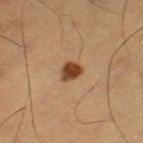follow-up = imaged on a skin check; not biopsied
location = the left thigh
image = 15 mm crop, total-body photography
illumination = cross-polarized illumination
patient = male, aged 58 to 62
automated metrics = a shape eccentricity near 0.6 and two-axis asymmetry of about 0.3; lesion-presence confidence of about 100/100
lesion diameter = about 2.5 mm